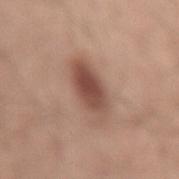{
  "biopsy_status": "not biopsied; imaged during a skin examination",
  "lighting": "white-light",
  "image": {
    "source": "total-body photography crop",
    "field_of_view_mm": 15
  },
  "site": "lower back",
  "patient": {
    "sex": "male",
    "age_approx": 30
  },
  "automated_metrics": {
    "area_mm2_approx": 12.0,
    "eccentricity": 0.8,
    "shape_asymmetry": 0.15,
    "cielab_L": 50,
    "cielab_a": 21,
    "cielab_b": 26,
    "vs_skin_contrast_norm": 9.0,
    "border_irregularity_0_10": 1.5,
    "color_variation_0_10": 5.0,
    "peripheral_color_asymmetry": 1.5,
    "lesion_detection_confidence_0_100": 100
  },
  "lesion_size": {
    "long_diameter_mm_approx": 5.0
  }
}